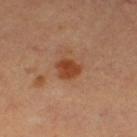The lesion is on the leg. Captured under cross-polarized illumination. The subject is a female aged approximately 30. A region of skin cropped from a whole-body photographic capture, roughly 15 mm wide. Approximately 3 mm at its widest. The total-body-photography lesion software estimated a lesion area of about 6 mm² and two-axis asymmetry of about 0.25. And it measured a border-irregularity rating of about 2/10 and a within-lesion color-variation index near 2.5/10.